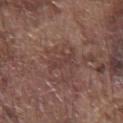Clinical impression:
No biopsy was performed on this lesion — it was imaged during a full skin examination and was not determined to be concerning.
Context:
The lesion is on the front of the torso. Longest diameter approximately 4 mm. A male patient, about 75 years old. A close-up tile cropped from a whole-body skin photograph, about 15 mm across. The tile uses white-light illumination.